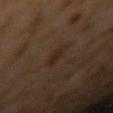location: the right upper arm; patient: male, aged 58–62; image source: total-body-photography crop, ~15 mm field of view; diameter: ~2.5 mm (longest diameter); tile lighting: cross-polarized illumination.